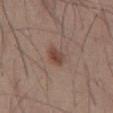Assessment: Imaged during a routine full-body skin examination; the lesion was not biopsied and no histopathology is available. Clinical summary: Located on the abdomen. A 15 mm crop from a total-body photograph taken for skin-cancer surveillance. A male patient, roughly 55 years of age.